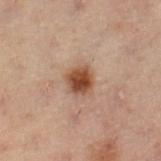Findings:
- follow-up · imaged on a skin check; not biopsied
- patient · female, approximately 55 years of age
- imaging modality · total-body-photography crop, ~15 mm field of view
- lighting · cross-polarized
- lesion diameter · about 3 mm
- anatomic site · the left leg
- automated lesion analysis · about 12 CIELAB-L* units darker than the surrounding skin and a normalized lesion–skin contrast near 11; a border-irregularity rating of about 1.5/10, a color-variation rating of about 4/10, and peripheral color asymmetry of about 1; an automated nevus-likeness rating near 100 out of 100 and lesion-presence confidence of about 100/100